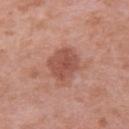Q: Is there a histopathology result?
A: imaged on a skin check; not biopsied
Q: Automated lesion metrics?
A: an area of roughly 11 mm², an eccentricity of roughly 0.55, and two-axis asymmetry of about 0.2; roughly 10 lightness units darker than nearby skin; a lesion-detection confidence of about 100/100
Q: What kind of image is this?
A: total-body-photography crop, ~15 mm field of view
Q: What is the anatomic site?
A: the left upper arm
Q: How was the tile lit?
A: white-light
Q: What are the patient's age and sex?
A: female, in their 40s
Q: Lesion size?
A: ~4 mm (longest diameter)